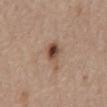Assessment: Recorded during total-body skin imaging; not selected for excision or biopsy. Acquisition and patient details: Automated image analysis of the tile measured an average lesion color of about L≈49 a*≈18 b*≈28 (CIELAB) and a normalized border contrast of about 9. And it measured a within-lesion color-variation index near 10/10 and a peripheral color-asymmetry measure near 3.5. The analysis additionally found a classifier nevus-likeness of about 90/100 and lesion-presence confidence of about 100/100. A male patient, aged 63 to 67. The lesion is on the front of the torso. Cropped from a total-body skin-imaging series; the visible field is about 15 mm. The recorded lesion diameter is about 3.5 mm.